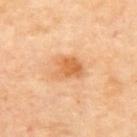workup = imaged on a skin check; not biopsied | anatomic site = the upper back | lesion diameter = about 3.5 mm | subject = female, aged 38 to 42 | image = ~15 mm tile from a whole-body skin photo | lighting = cross-polarized.